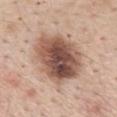Notes:
– anatomic site — the back
– patient — male, approximately 55 years of age
– tile lighting — white-light
– TBP lesion metrics — a mean CIELAB color near L≈52 a*≈19 b*≈26 and about 17 CIELAB-L* units darker than the surrounding skin; a detector confidence of about 100 out of 100 that the crop contains a lesion
– image — ~15 mm tile from a whole-body skin photo
– size — ~6.5 mm (longest diameter)
– biopsy diagnosis — a dysplastic (Clark) nevus